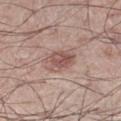The lesion was tiled from a total-body skin photograph and was not biopsied. The lesion's longest dimension is about 3 mm. Cropped from a total-body skin-imaging series; the visible field is about 15 mm. A male subject, about 60 years old. From the right thigh. The lesion-visualizer software estimated an average lesion color of about L≈53 a*≈20 b*≈23 (CIELAB), roughly 10 lightness units darker than nearby skin, and a lesion-to-skin contrast of about 7 (normalized; higher = more distinct). The software also gave a classifier nevus-likeness of about 80/100 and a detector confidence of about 100 out of 100 that the crop contains a lesion. The tile uses white-light illumination.